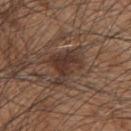subject: male, roughly 45 years of age | size: about 4.5 mm | acquisition: ~15 mm tile from a whole-body skin photo | automated metrics: an area of roughly 10 mm², an eccentricity of roughly 0.55, and two-axis asymmetry of about 0.5 | site: the upper back.